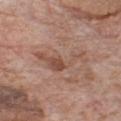Q: Was a biopsy performed?
A: no biopsy performed (imaged during a skin exam)
Q: Automated lesion metrics?
A: about 8 CIELAB-L* units darker than the surrounding skin and a lesion-to-skin contrast of about 6 (normalized; higher = more distinct); an automated nevus-likeness rating near 0 out of 100 and a lesion-detection confidence of about 100/100
Q: Lesion size?
A: about 6 mm
Q: What lighting was used for the tile?
A: white-light
Q: What kind of image is this?
A: 15 mm crop, total-body photography
Q: Patient demographics?
A: male, aged 78 to 82
Q: Lesion location?
A: the chest